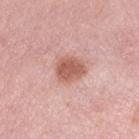<case>
<site>left thigh</site>
<lighting>white-light</lighting>
<patient>
  <sex>female</sex>
  <age_approx>50</age_approx>
</patient>
<automated_metrics>
  <cielab_L>59</cielab_L>
  <cielab_a>24</cielab_a>
  <cielab_b>28</cielab_b>
  <vs_skin_darker_L>12.0</vs_skin_darker_L>
  <vs_skin_contrast_norm>8.5</vs_skin_contrast_norm>
  <border_irregularity_0_10>1.5</border_irregularity_0_10>
  <color_variation_0_10>2.5</color_variation_0_10>
  <peripheral_color_asymmetry>1.0</peripheral_color_asymmetry>
  <nevus_likeness_0_100>85</nevus_likeness_0_100>
  <lesion_detection_confidence_0_100>100</lesion_detection_confidence_0_100>
</automated_metrics>
<lesion_size>
  <long_diameter_mm_approx>3.5</long_diameter_mm_approx>
</lesion_size>
<image>
  <source>total-body photography crop</source>
  <field_of_view_mm>15</field_of_view_mm>
</image>
</case>